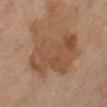Context: A female subject, aged 68–72. The recorded lesion diameter is about 8 mm. This image is a 15 mm lesion crop taken from a total-body photograph. The lesion is located on the leg.